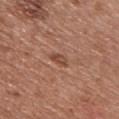The lesion was photographed on a routine skin check and not biopsied; there is no pathology result. This is a white-light tile. A male patient in their mid-50s. The lesion is located on the front of the torso. The total-body-photography lesion software estimated a lesion color around L≈47 a*≈22 b*≈29 in CIELAB and a lesion-to-skin contrast of about 6.5 (normalized; higher = more distinct). A 15 mm close-up extracted from a 3D total-body photography capture.